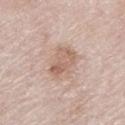biopsy_status: not biopsied; imaged during a skin examination
lesion_size:
  long_diameter_mm_approx: 4.0
automated_metrics:
  area_mm2_approx: 8.5
  eccentricity: 0.8
  shape_asymmetry: 0.3
  cielab_L: 62
  cielab_a: 18
  cielab_b: 27
  vs_skin_darker_L: 9.0
  vs_skin_contrast_norm: 6.5
  color_variation_0_10: 5.0
  peripheral_color_asymmetry: 1.5
  nevus_likeness_0_100: 10
  lesion_detection_confidence_0_100: 100
lighting: white-light
patient:
  sex: male
  age_approx: 80
image:
  source: total-body photography crop
  field_of_view_mm: 15
site: left lower leg